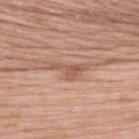Captured during whole-body skin photography for melanoma surveillance; the lesion was not biopsied. Longest diameter approximately 4.5 mm. Automated tile analysis of the lesion measured a footprint of about 5.5 mm², a shape eccentricity near 0.9, and a symmetry-axis asymmetry near 0.6. The software also gave an average lesion color of about L≈56 a*≈21 b*≈29 (CIELAB), about 9 CIELAB-L* units darker than the surrounding skin, and a lesion-to-skin contrast of about 6.5 (normalized; higher = more distinct). The software also gave a border-irregularity index near 7/10, a within-lesion color-variation index near 2/10, and a peripheral color-asymmetry measure near 0.5. The analysis additionally found a nevus-likeness score of about 0/100 and lesion-presence confidence of about 95/100. From the upper back. A female patient approximately 60 years of age. Captured under white-light illumination. A 15 mm close-up extracted from a 3D total-body photography capture.